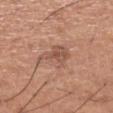No biopsy was performed on this lesion — it was imaged during a full skin examination and was not determined to be concerning.
Located on the left thigh.
The patient is a male aged approximately 65.
A close-up tile cropped from a whole-body skin photograph, about 15 mm across.